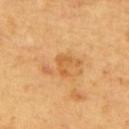Assessment:
Captured during whole-body skin photography for melanoma surveillance; the lesion was not biopsied.
Background:
The tile uses cross-polarized illumination. Cropped from a whole-body photographic skin survey; the tile spans about 15 mm. Approximately 2.5 mm at its widest. The lesion is on the upper back. A male subject, aged around 65. Automated tile analysis of the lesion measured an area of roughly 3 mm² and a symmetry-axis asymmetry near 0.45. And it measured a lesion color around L≈60 a*≈24 b*≈46 in CIELAB and a lesion-to-skin contrast of about 5.5 (normalized; higher = more distinct). It also reported a border-irregularity index near 4.5/10, a within-lesion color-variation index near 1/10, and radial color variation of about 0.5. And it measured an automated nevus-likeness rating near 0 out of 100 and a lesion-detection confidence of about 100/100.